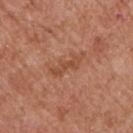Imaged with white-light lighting. Automated tile analysis of the lesion measured a lesion area of about 4 mm², a shape eccentricity near 0.95, and a shape-asymmetry score of about 0.35 (0 = symmetric). The analysis additionally found a mean CIELAB color near L≈48 a*≈24 b*≈32. And it measured a within-lesion color-variation index near 0/10. It also reported a classifier nevus-likeness of about 0/100 and a detector confidence of about 100 out of 100 that the crop contains a lesion. A close-up tile cropped from a whole-body skin photograph, about 15 mm across. A male subject, aged 53–57. Approximately 4 mm at its widest. The lesion is located on the chest.